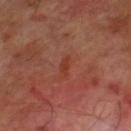Clinical impression:
This lesion was catalogued during total-body skin photography and was not selected for biopsy.
Background:
A lesion tile, about 15 mm wide, cut from a 3D total-body photograph. On the right lower leg. A male subject aged 68–72. The tile uses cross-polarized illumination. Measured at roughly 2.5 mm in maximum diameter.